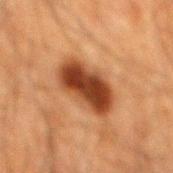Captured during whole-body skin photography for melanoma surveillance; the lesion was not biopsied.
From the mid back.
A lesion tile, about 15 mm wide, cut from a 3D total-body photograph.
The patient is a male aged around 60.